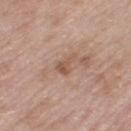The lesion was tiled from a total-body skin photograph and was not biopsied.
From the leg.
A roughly 15 mm field-of-view crop from a total-body skin photograph.
Automated image analysis of the tile measured a lesion area of about 3.5 mm², an outline eccentricity of about 0.75 (0 = round, 1 = elongated), and a symmetry-axis asymmetry near 0.45. The software also gave a border-irregularity index near 4.5/10, internal color variation of about 2.5 on a 0–10 scale, and radial color variation of about 1.
The subject is a female aged 68–72.